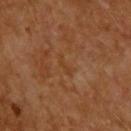Q: Was this lesion biopsied?
A: no biopsy performed (imaged during a skin exam)
Q: Who is the patient?
A: male, aged 58–62
Q: What kind of image is this?
A: total-body-photography crop, ~15 mm field of view
Q: What did automated image analysis measure?
A: a footprint of about 2.5 mm² and an outline eccentricity of about 0.9 (0 = round, 1 = elongated); an automated nevus-likeness rating near 0 out of 100 and a detector confidence of about 100 out of 100 that the crop contains a lesion
Q: Illumination type?
A: cross-polarized
Q: What is the anatomic site?
A: the upper back
Q: How large is the lesion?
A: ~3 mm (longest diameter)